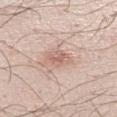Acquisition and patient details: A 15 mm close-up extracted from a 3D total-body photography capture. The subject is a male aged approximately 25. Measured at roughly 2.5 mm in maximum diameter. From the left lower leg.